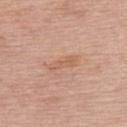| field | value |
|---|---|
| follow-up | total-body-photography surveillance lesion; no biopsy |